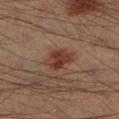biopsy_status: not biopsied; imaged during a skin examination
patient:
  sex: male
  age_approx: 40
site: left lower leg
lesion_size:
  long_diameter_mm_approx: 3.5
image:
  source: total-body photography crop
  field_of_view_mm: 15
lighting: cross-polarized